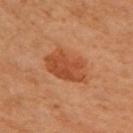Case summary:
• workup: imaged on a skin check; not biopsied
• site: the right arm
• illumination: cross-polarized
• size: about 5 mm
• automated metrics: an average lesion color of about L≈42 a*≈25 b*≈34 (CIELAB) and about 9 CIELAB-L* units darker than the surrounding skin; border irregularity of about 2 on a 0–10 scale and peripheral color asymmetry of about 1; a classifier nevus-likeness of about 90/100 and lesion-presence confidence of about 100/100
• patient: female, aged approximately 60
• acquisition: total-body-photography crop, ~15 mm field of view